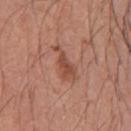Context:
The lesion is located on the upper back. The lesion's longest dimension is about 4.5 mm. A lesion tile, about 15 mm wide, cut from a 3D total-body photograph. This is a white-light tile. The lesion-visualizer software estimated a nevus-likeness score of about 60/100 and a lesion-detection confidence of about 100/100. The subject is a male in their mid-40s.